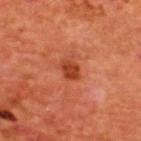Q: Where on the body is the lesion?
A: the upper back
Q: What kind of image is this?
A: ~15 mm crop, total-body skin-cancer survey
Q: What are the patient's age and sex?
A: male, approximately 65 years of age
Q: How was the tile lit?
A: cross-polarized
Q: What is the lesion's diameter?
A: ~2.5 mm (longest diameter)
Q: What did automated image analysis measure?
A: a classifier nevus-likeness of about 75/100 and a detector confidence of about 100 out of 100 that the crop contains a lesion